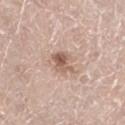notes = total-body-photography surveillance lesion; no biopsy
image = ~15 mm crop, total-body skin-cancer survey
subject = male, in their 70s
location = the right leg